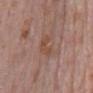– notes — imaged on a skin check; not biopsied